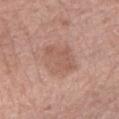An algorithmic analysis of the crop reported a lesion area of about 14 mm² and a shape-asymmetry score of about 0.15 (0 = symmetric). The software also gave a mean CIELAB color near L≈57 a*≈20 b*≈27 and a normalized border contrast of about 5. A female subject roughly 40 years of age. The recorded lesion diameter is about 4.5 mm. A 15 mm close-up tile from a total-body photography series done for melanoma screening. Imaged with white-light lighting. The lesion is located on the right forearm.